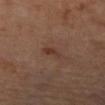  biopsy_status: not biopsied; imaged during a skin examination
  patient:
    sex: female
    age_approx: 65
  lighting: cross-polarized
  automated_metrics:
    cielab_L: 35
    cielab_a: 19
    cielab_b: 25
    vs_skin_darker_L: 7.0
    border_irregularity_0_10: 4.0
    color_variation_0_10: 0.5
    peripheral_color_asymmetry: 0.0
  site: left forearm
  image:
    source: total-body photography crop
    field_of_view_mm: 15
  lesion_size:
    long_diameter_mm_approx: 2.5